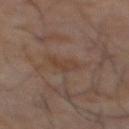biopsy_status: not biopsied; imaged during a skin examination
patient:
  sex: male
  age_approx: 60
lesion_size:
  long_diameter_mm_approx: 3.0
site: abdomen
image:
  source: total-body photography crop
  field_of_view_mm: 15
lighting: cross-polarized